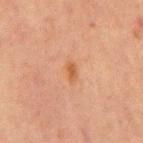Clinical impression: Imaged during a routine full-body skin examination; the lesion was not biopsied and no histopathology is available. Image and clinical context: The lesion's longest dimension is about 2.5 mm. The lesion-visualizer software estimated a shape eccentricity near 0.85 and a symmetry-axis asymmetry near 0.25. And it measured about 6 CIELAB-L* units darker than the surrounding skin and a lesion-to-skin contrast of about 6.5 (normalized; higher = more distinct). And it measured a classifier nevus-likeness of about 80/100 and a detector confidence of about 100 out of 100 that the crop contains a lesion. The lesion is located on the chest. A male subject aged approximately 65. This is a cross-polarized tile. This image is a 15 mm lesion crop taken from a total-body photograph.